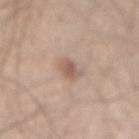Q: Is there a histopathology result?
A: catalogued during a skin exam; not biopsied
Q: What is the imaging modality?
A: ~15 mm tile from a whole-body skin photo
Q: What lighting was used for the tile?
A: white-light illumination
Q: Where on the body is the lesion?
A: the back
Q: What are the patient's age and sex?
A: male, about 45 years old
Q: How large is the lesion?
A: ~2.5 mm (longest diameter)